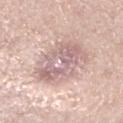On the left lower leg. This is a white-light tile. Longest diameter approximately 6 mm. This image is a 15 mm lesion crop taken from a total-body photograph. A male subject aged 58–62.